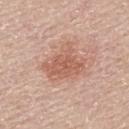  site: upper back
  image:
    source: total-body photography crop
    field_of_view_mm: 15
  patient:
    sex: male
    age_approx: 60
  automated_metrics:
    eccentricity: 0.6
    shape_asymmetry: 0.35
    cielab_L: 59
    cielab_a: 23
    cielab_b: 29
    vs_skin_darker_L: 9.0
    vs_skin_contrast_norm: 6.5
    border_irregularity_0_10: 4.0
    color_variation_0_10: 3.0
    peripheral_color_asymmetry: 1.0
  lesion_size:
    long_diameter_mm_approx: 5.0
  lighting: white-light